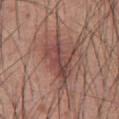Case summary:
- biopsy status: catalogued during a skin exam; not biopsied
- body site: the abdomen
- patient: male, aged approximately 60
- image-analysis metrics: an area of roughly 15 mm²; an average lesion color of about L≈45 a*≈22 b*≈22 (CIELAB), about 10 CIELAB-L* units darker than the surrounding skin, and a normalized lesion–skin contrast near 7.5; a color-variation rating of about 7/10 and peripheral color asymmetry of about 2.5
- imaging modality: total-body-photography crop, ~15 mm field of view
- lesion diameter: ~6.5 mm (longest diameter)
- tile lighting: white-light